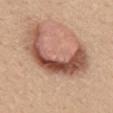The lesion was tiled from a total-body skin photograph and was not biopsied. This is a white-light tile. The recorded lesion diameter is about 9.5 mm. The lesion is located on the mid back. Cropped from a whole-body photographic skin survey; the tile spans about 15 mm. Automated image analysis of the tile measured an area of roughly 24 mm². The software also gave an average lesion color of about L≈52 a*≈22 b*≈29 (CIELAB), about 16 CIELAB-L* units darker than the surrounding skin, and a normalized lesion–skin contrast near 10.5. It also reported a nevus-likeness score of about 80/100 and a lesion-detection confidence of about 15/100. A female patient, roughly 35 years of age.